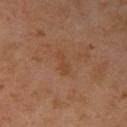Impression: The lesion was tiled from a total-body skin photograph and was not biopsied. Image and clinical context: A female patient aged approximately 55. A 15 mm close-up extracted from a 3D total-body photography capture. Imaged with cross-polarized lighting. Located on the right upper arm. Approximately 3 mm at its widest.